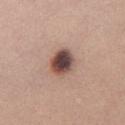biopsy status: no biopsy performed (imaged during a skin exam)
subject: female, in their mid- to late 50s
acquisition: ~15 mm crop, total-body skin-cancer survey
diameter: ≈3.5 mm
illumination: white-light illumination
automated lesion analysis: a footprint of about 8.5 mm²; a border-irregularity rating of about 1.5/10, internal color variation of about 8.5 on a 0–10 scale, and a peripheral color-asymmetry measure near 2.5; a classifier nevus-likeness of about 70/100 and lesion-presence confidence of about 100/100
anatomic site: the chest